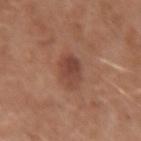Assessment:
No biopsy was performed on this lesion — it was imaged during a full skin examination and was not determined to be concerning.
Context:
Imaged with white-light lighting. A lesion tile, about 15 mm wide, cut from a 3D total-body photograph. A female subject aged approximately 40. From the right upper arm.